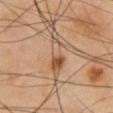This lesion was catalogued during total-body skin photography and was not selected for biopsy. Located on the chest. The patient is a male aged 53–57. This image is a 15 mm lesion crop taken from a total-body photograph. The lesion's longest dimension is about 5 mm. The total-body-photography lesion software estimated an area of roughly 7 mm² and a shape-asymmetry score of about 0.4 (0 = symmetric). And it measured a lesion color around L≈43 a*≈15 b*≈27 in CIELAB, about 8 CIELAB-L* units darker than the surrounding skin, and a lesion-to-skin contrast of about 6.5 (normalized; higher = more distinct). And it measured a border-irregularity index near 5/10, internal color variation of about 7 on a 0–10 scale, and radial color variation of about 2.